The lesion is located on the head or neck, longest diameter approximately 1.5 mm, the patient is a male in their mid-50s, imaged with white-light lighting, Automated tile analysis of the lesion measured a border-irregularity rating of about 3.5/10 and a peripheral color-asymmetry measure near 0, a 15 mm close-up extracted from a 3D total-body photography capture.
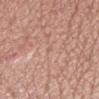Notes:
• histopathologic diagnosis · an intradermal melanocytic nevus